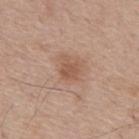{
  "biopsy_status": "not biopsied; imaged during a skin examination",
  "lesion_size": {
    "long_diameter_mm_approx": 2.5
  },
  "automated_metrics": {
    "cielab_L": 54,
    "cielab_a": 20,
    "cielab_b": 30,
    "vs_skin_darker_L": 8.0,
    "vs_skin_contrast_norm": 6.0
  },
  "image": {
    "source": "total-body photography crop",
    "field_of_view_mm": 15
  },
  "patient": {
    "sex": "male",
    "age_approx": 55
  }
}